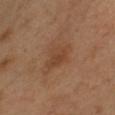notes: no biopsy performed (imaged during a skin exam)
imaging modality: 15 mm crop, total-body photography
automated lesion analysis: a footprint of about 6 mm², an eccentricity of roughly 0.8, and a symmetry-axis asymmetry near 0.3; a mean CIELAB color near L≈43 a*≈20 b*≈33, roughly 7 lightness units darker than nearby skin, and a lesion-to-skin contrast of about 6 (normalized; higher = more distinct); a color-variation rating of about 2.5/10 and radial color variation of about 0.5
anatomic site: the chest
subject: female, aged approximately 40